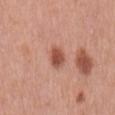Impression:
No biopsy was performed on this lesion — it was imaged during a full skin examination and was not determined to be concerning.
Image and clinical context:
The lesion's longest dimension is about 2.5 mm. Cropped from a whole-body photographic skin survey; the tile spans about 15 mm. The total-body-photography lesion software estimated a lesion area of about 4.5 mm², an outline eccentricity of about 0.65 (0 = round, 1 = elongated), and a symmetry-axis asymmetry near 0.2. The software also gave roughly 13 lightness units darker than nearby skin and a lesion-to-skin contrast of about 9 (normalized; higher = more distinct). It also reported a border-irregularity rating of about 2/10, a within-lesion color-variation index near 3.5/10, and peripheral color asymmetry of about 1. The lesion is on the mid back. The subject is a male aged approximately 70.